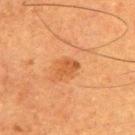Context: A close-up tile cropped from a whole-body skin photograph, about 15 mm across. About 3 mm across. A male subject, aged around 50. The tile uses cross-polarized illumination. From the back. Automated image analysis of the tile measured an area of roughly 4 mm² and a shape-asymmetry score of about 0.3 (0 = symmetric). The analysis additionally found an average lesion color of about L≈45 a*≈24 b*≈37 (CIELAB). The analysis additionally found a border-irregularity index near 3/10, a within-lesion color-variation index near 2/10, and a peripheral color-asymmetry measure near 0.5. The analysis additionally found a nevus-likeness score of about 25/100.